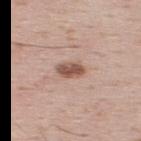Acquisition and patient details: A male subject aged approximately 35. A roughly 15 mm field-of-view crop from a total-body skin photograph. The lesion's longest dimension is about 3 mm. On the upper back.